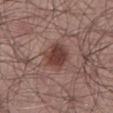Automated image analysis of the tile measured a lesion area of about 9 mm² and a shape-asymmetry score of about 0.15 (0 = symmetric). It also reported a nevus-likeness score of about 95/100 and a detector confidence of about 100 out of 100 that the crop contains a lesion. Captured under white-light illumination. Longest diameter approximately 3.5 mm. A lesion tile, about 15 mm wide, cut from a 3D total-body photograph. A male subject, in their mid- to late 50s. Located on the left lower leg.